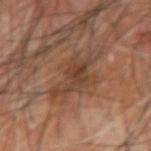follow-up: catalogued during a skin exam; not biopsied | body site: the left forearm | size: ~7 mm (longest diameter) | subject: male, in their mid- to late 60s | automated lesion analysis: lesion-presence confidence of about 80/100 | acquisition: 15 mm crop, total-body photography.